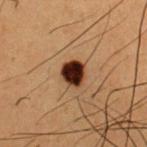Q: Was a biopsy performed?
A: catalogued during a skin exam; not biopsied
Q: Patient demographics?
A: male, aged 48–52
Q: Lesion size?
A: about 3 mm
Q: What lighting was used for the tile?
A: cross-polarized illumination
Q: How was this image acquired?
A: 15 mm crop, total-body photography
Q: Where on the body is the lesion?
A: the chest
Q: What did automated image analysis measure?
A: an average lesion color of about L≈23 a*≈18 b*≈23 (CIELAB) and a normalized lesion–skin contrast near 20; a border-irregularity index near 2/10, a color-variation rating of about 4.5/10, and peripheral color asymmetry of about 1.5